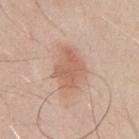{
  "site": "mid back",
  "lighting": "white-light",
  "patient": {
    "sex": "male",
    "age_approx": 50
  },
  "lesion_size": {
    "long_diameter_mm_approx": 5.0
  },
  "image": {
    "source": "total-body photography crop",
    "field_of_view_mm": 15
  }
}